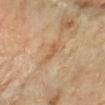The lesion was tiled from a total-body skin photograph and was not biopsied. From the left forearm. Cropped from a total-body skin-imaging series; the visible field is about 15 mm. A female subject aged 58 to 62. Imaged with cross-polarized lighting. Measured at roughly 2.5 mm in maximum diameter.